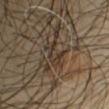The lesion was tiled from a total-body skin photograph and was not biopsied.
A male patient, aged 43 to 47.
Imaged with cross-polarized lighting.
Approximately 3.5 mm at its widest.
The lesion is on the left upper arm.
A 15 mm close-up tile from a total-body photography series done for melanoma screening.
The lesion-visualizer software estimated a lesion area of about 6.5 mm², an eccentricity of roughly 0.75, and a symmetry-axis asymmetry near 0.4.